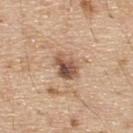Assessment: Part of a total-body skin-imaging series; this lesion was reviewed on a skin check and was not flagged for biopsy. Image and clinical context: A male subject about 70 years old. Cropped from a total-body skin-imaging series; the visible field is about 15 mm. Located on the back.